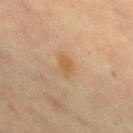{
  "biopsy_status": "not biopsied; imaged during a skin examination",
  "site": "chest",
  "lesion_size": {
    "long_diameter_mm_approx": 3.0
  },
  "automated_metrics": {
    "border_irregularity_0_10": 2.0,
    "color_variation_0_10": 1.5,
    "peripheral_color_asymmetry": 0.5
  },
  "image": {
    "source": "total-body photography crop",
    "field_of_view_mm": 15
  },
  "lighting": "cross-polarized",
  "patient": {
    "sex": "female",
    "age_approx": 60
  }
}